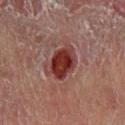Q: Was a biopsy performed?
A: total-body-photography surveillance lesion; no biopsy
Q: How was this image acquired?
A: ~15 mm tile from a whole-body skin photo
Q: Lesion location?
A: the leg
Q: Patient demographics?
A: male, roughly 55 years of age
Q: Automated lesion metrics?
A: a footprint of about 11 mm², an outline eccentricity of about 0.75 (0 = round, 1 = elongated), and a symmetry-axis asymmetry near 0.25; a lesion color around L≈30 a*≈25 b*≈22 in CIELAB, roughly 13 lightness units darker than nearby skin, and a normalized lesion–skin contrast near 12; border irregularity of about 2.5 on a 0–10 scale and internal color variation of about 5 on a 0–10 scale; an automated nevus-likeness rating near 5 out of 100 and a detector confidence of about 100 out of 100 that the crop contains a lesion
Q: Illumination type?
A: cross-polarized illumination
Q: How large is the lesion?
A: about 4.5 mm